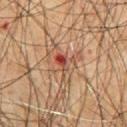Part of a total-body skin-imaging series; this lesion was reviewed on a skin check and was not flagged for biopsy. The lesion is on the abdomen. A male subject aged 63 to 67. Automated image analysis of the tile measured a footprint of about 11 mm², an eccentricity of roughly 0.55, and two-axis asymmetry of about 0.45. It also reported a lesion color around L≈43 a*≈19 b*≈27 in CIELAB and a normalized border contrast of about 6.5. The software also gave a border-irregularity index near 6/10 and a peripheral color-asymmetry measure near 5.5. Captured under cross-polarized illumination. The lesion's longest dimension is about 4.5 mm. A 15 mm crop from a total-body photograph taken for skin-cancer surveillance.